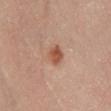This is a cross-polarized tile.
An algorithmic analysis of the crop reported a lesion area of about 4 mm², an outline eccentricity of about 0.35 (0 = round, 1 = elongated), and a shape-asymmetry score of about 0.2 (0 = symmetric). The software also gave a classifier nevus-likeness of about 95/100 and lesion-presence confidence of about 100/100.
The patient is a female aged 63 to 67.
The lesion's longest dimension is about 2 mm.
From the right lower leg.
A close-up tile cropped from a whole-body skin photograph, about 15 mm across.